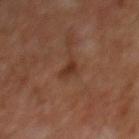{
  "biopsy_status": "not biopsied; imaged during a skin examination",
  "automated_metrics": {
    "cielab_L": 30,
    "cielab_a": 19,
    "cielab_b": 26,
    "vs_skin_darker_L": 7.0,
    "vs_skin_contrast_norm": 7.0,
    "peripheral_color_asymmetry": 0.5
  },
  "lesion_size": {
    "long_diameter_mm_approx": 2.5
  },
  "patient": {
    "sex": "male",
    "age_approx": 60
  },
  "site": "mid back",
  "lighting": "cross-polarized",
  "image": {
    "source": "total-body photography crop",
    "field_of_view_mm": 15
  }
}